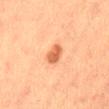Part of a total-body skin-imaging series; this lesion was reviewed on a skin check and was not flagged for biopsy. This is a cross-polarized tile. A female subject in their mid- to late 50s. About 2.5 mm across. Located on the lower back. A 15 mm crop from a total-body photograph taken for skin-cancer surveillance.